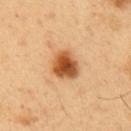Assessment: Captured during whole-body skin photography for melanoma surveillance; the lesion was not biopsied. Acquisition and patient details: On the abdomen. Automated image analysis of the tile measured about 17 CIELAB-L* units darker than the surrounding skin and a normalized lesion–skin contrast near 11.5. The analysis additionally found a border-irregularity index near 2/10, a color-variation rating of about 6/10, and a peripheral color-asymmetry measure near 1.5. And it measured lesion-presence confidence of about 100/100. The subject is a male in their 50s. Cropped from a whole-body photographic skin survey; the tile spans about 15 mm. Longest diameter approximately 4 mm.